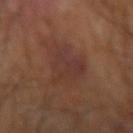follow-up = imaged on a skin check; not biopsied | image source = 15 mm crop, total-body photography | anatomic site = the right forearm | tile lighting = cross-polarized.